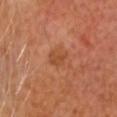Part of a total-body skin-imaging series; this lesion was reviewed on a skin check and was not flagged for biopsy. A region of skin cropped from a whole-body photographic capture, roughly 15 mm wide. The lesion's longest dimension is about 3 mm. This is a cross-polarized tile. A male subject approximately 65 years of age. Automated tile analysis of the lesion measured a footprint of about 4 mm², an eccentricity of roughly 0.8, and a symmetry-axis asymmetry near 0.3. The analysis additionally found a border-irregularity rating of about 3/10 and internal color variation of about 1.5 on a 0–10 scale. The analysis additionally found lesion-presence confidence of about 100/100.